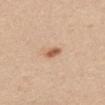The lesion was tiled from a total-body skin photograph and was not biopsied.
A close-up tile cropped from a whole-body skin photograph, about 15 mm across.
Located on the upper back.
A female patient aged 28–32.